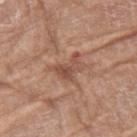Notes:
* notes — no biopsy performed (imaged during a skin exam)
* image-analysis metrics — border irregularity of about 6.5 on a 0–10 scale and internal color variation of about 2 on a 0–10 scale; a classifier nevus-likeness of about 5/100 and a detector confidence of about 100 out of 100 that the crop contains a lesion
* image — ~15 mm crop, total-body skin-cancer survey
* tile lighting — white-light illumination
* site — the right thigh
* subject — female, aged around 75